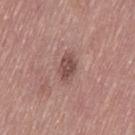Q: Was this lesion biopsied?
A: imaged on a skin check; not biopsied
Q: What is the anatomic site?
A: the left thigh
Q: Lesion size?
A: about 3 mm
Q: Illumination type?
A: white-light illumination
Q: What are the patient's age and sex?
A: female, roughly 65 years of age
Q: What is the imaging modality?
A: total-body-photography crop, ~15 mm field of view